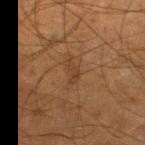Impression: Recorded during total-body skin imaging; not selected for excision or biopsy. Acquisition and patient details: Imaged with cross-polarized lighting. Cropped from a total-body skin-imaging series; the visible field is about 15 mm. Longest diameter approximately 3 mm. A male subject aged approximately 65. From the leg.